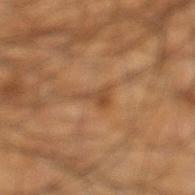- subject — male, aged 43–47
- image — total-body-photography crop, ~15 mm field of view
- location — the leg